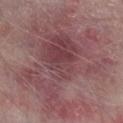<tbp_lesion>
  <biopsy_status>not biopsied; imaged during a skin examination</biopsy_status>
  <site>right lower leg</site>
  <lesion_size>
    <long_diameter_mm_approx>13.5</long_diameter_mm_approx>
  </lesion_size>
  <lighting>white-light</lighting>
  <automated_metrics>
    <cielab_L>47</cielab_L>
    <cielab_a>22</cielab_a>
    <cielab_b>17</cielab_b>
    <vs_skin_darker_L>9.0</vs_skin_darker_L>
    <vs_skin_contrast_norm>7.0</vs_skin_contrast_norm>
    <border_irregularity_0_10>8.0</border_irregularity_0_10>
    <peripheral_color_asymmetry>2.0</peripheral_color_asymmetry>
  </automated_metrics>
  <patient>
    <sex>male</sex>
    <age_approx>75</age_approx>
  </patient>
  <image>
    <source>total-body photography crop</source>
    <field_of_view_mm>15</field_of_view_mm>
  </image>
</tbp_lesion>